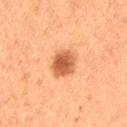diameter = about 4 mm | patient = female, about 50 years old | automated lesion analysis = an area of roughly 8.5 mm²; a mean CIELAB color near L≈49 a*≈24 b*≈35 and roughly 13 lightness units darker than nearby skin; border irregularity of about 1.5 on a 0–10 scale and a within-lesion color-variation index near 3.5/10; a nevus-likeness score of about 95/100 and a detector confidence of about 100 out of 100 that the crop contains a lesion | lighting = cross-polarized | anatomic site = the left thigh | image = 15 mm crop, total-body photography.